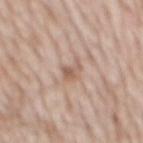The lesion is on the mid back. The lesion's longest dimension is about 3 mm. Cropped from a whole-body photographic skin survey; the tile spans about 15 mm. A male patient, roughly 65 years of age.